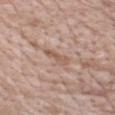Findings:
* notes — catalogued during a skin exam; not biopsied
* illumination — white-light
* TBP lesion metrics — a footprint of about 4 mm² and a shape-asymmetry score of about 0.35 (0 = symmetric); an average lesion color of about L≈57 a*≈18 b*≈27 (CIELAB) and about 8 CIELAB-L* units darker than the surrounding skin; a border-irregularity index near 3.5/10, internal color variation of about 1 on a 0–10 scale, and peripheral color asymmetry of about 0.5
* imaging modality — 15 mm crop, total-body photography
* patient — female, in their mid- to late 70s
* body site — the chest